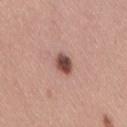The lesion is located on the lower back. The lesion's longest dimension is about 2.5 mm. The patient is a female aged 28 to 32. This image is a 15 mm lesion crop taken from a total-body photograph. The tile uses white-light illumination.